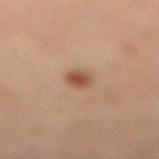Recorded during total-body skin imaging; not selected for excision or biopsy.
Located on the left leg.
Captured under cross-polarized illumination.
A roughly 15 mm field-of-view crop from a total-body skin photograph.
About 2.5 mm across.
The patient is a female aged 63 to 67.